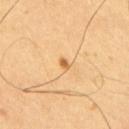<tbp_lesion>
<biopsy_status>not biopsied; imaged during a skin examination</biopsy_status>
<image>
  <source>total-body photography crop</source>
  <field_of_view_mm>15</field_of_view_mm>
</image>
<site>upper back</site>
<lesion_size>
  <long_diameter_mm_approx>1.5</long_diameter_mm_approx>
</lesion_size>
<lighting>cross-polarized</lighting>
<patient>
  <sex>male</sex>
  <age_approx>65</age_approx>
</patient>
<automated_metrics>
  <area_mm2_approx>1.5</area_mm2_approx>
  <shape_asymmetry>0.35</shape_asymmetry>
  <cielab_L>61</cielab_L>
  <cielab_a>22</cielab_a>
  <cielab_b>44</cielab_b>
  <vs_skin_darker_L>12.0</vs_skin_darker_L>
  <vs_skin_contrast_norm>8.0</vs_skin_contrast_norm>
  <nevus_likeness_0_100>85</nevus_likeness_0_100>
</automated_metrics>
</tbp_lesion>